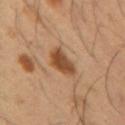Clinical impression: Captured during whole-body skin photography for melanoma surveillance; the lesion was not biopsied. Background: Measured at roughly 3 mm in maximum diameter. Imaged with cross-polarized lighting. The total-body-photography lesion software estimated an area of roughly 7 mm² and an eccentricity of roughly 0.5. It also reported a mean CIELAB color near L≈46 a*≈21 b*≈34, roughly 13 lightness units darker than nearby skin, and a normalized border contrast of about 9.5. This image is a 15 mm lesion crop taken from a total-body photograph. The lesion is on the left forearm. A male subject, approximately 40 years of age.